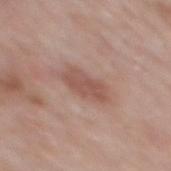| feature | finding |
|---|---|
| workup | imaged on a skin check; not biopsied |
| anatomic site | the mid back |
| TBP lesion metrics | a lesion–skin lightness drop of about 9 and a normalized lesion–skin contrast near 6.5; a border-irregularity rating of about 2.5/10 |
| imaging modality | total-body-photography crop, ~15 mm field of view |
| tile lighting | white-light |
| lesion size | ≈4.5 mm |
| patient | male, in their mid- to late 50s |